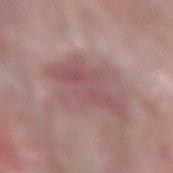patient:
  sex: male
  age_approx: 75
site: arm
lighting: white-light
image:
  source: total-body photography crop
  field_of_view_mm: 15
lesion_size:
  long_diameter_mm_approx: 7.0